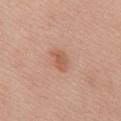Case summary:
• biopsy status · catalogued during a skin exam; not biopsied
• diameter · ≈3 mm
• illumination · white-light
• location · the front of the torso
• imaging modality · ~15 mm crop, total-body skin-cancer survey
• subject · female, roughly 65 years of age